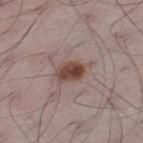notes — catalogued during a skin exam; not biopsied | lesion size — ≈4 mm | tile lighting — white-light illumination | anatomic site — the leg | subject — male, aged around 70 | acquisition — total-body-photography crop, ~15 mm field of view.